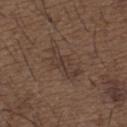workup: no biopsy performed (imaged during a skin exam) | body site: the upper back | lighting: white-light illumination | diameter: ~5.5 mm (longest diameter) | automated metrics: an average lesion color of about L≈37 a*≈14 b*≈23 (CIELAB), about 5 CIELAB-L* units darker than the surrounding skin, and a normalized border contrast of about 5.5; radial color variation of about 1; a nevus-likeness score of about 0/100 and lesion-presence confidence of about 90/100 | subject: male, approximately 50 years of age | image source: 15 mm crop, total-body photography.